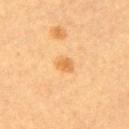biopsy status: catalogued during a skin exam; not biopsied
tile lighting: cross-polarized
subject: female, roughly 60 years of age
automated lesion analysis: a normalized lesion–skin contrast near 6.5; a border-irregularity index near 2/10; a classifier nevus-likeness of about 75/100 and a lesion-detection confidence of about 100/100
location: the upper back
lesion diameter: ≈2.5 mm
image: ~15 mm tile from a whole-body skin photo